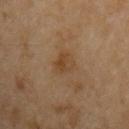Q: Was this lesion biopsied?
A: no biopsy performed (imaged during a skin exam)
Q: Who is the patient?
A: male, aged 53–57
Q: How was this image acquired?
A: ~15 mm tile from a whole-body skin photo
Q: What is the anatomic site?
A: the left upper arm